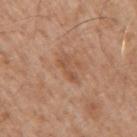follow-up — imaged on a skin check; not biopsied